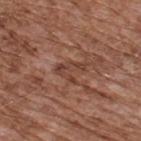Impression:
Imaged during a routine full-body skin examination; the lesion was not biopsied and no histopathology is available.